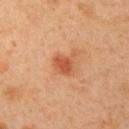This lesion was catalogued during total-body skin photography and was not selected for biopsy. Automated image analysis of the tile measured a lesion area of about 6 mm², an eccentricity of roughly 0.4, and two-axis asymmetry of about 0.35. The analysis additionally found a lesion color around L≈57 a*≈29 b*≈38 in CIELAB and a lesion–skin lightness drop of about 10. From the left upper arm. Approximately 2.5 mm at its widest. This image is a 15 mm lesion crop taken from a total-body photograph. A female patient, approximately 40 years of age. Captured under cross-polarized illumination.